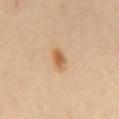No biopsy was performed on this lesion — it was imaged during a full skin examination and was not determined to be concerning. This image is a 15 mm lesion crop taken from a total-body photograph. This is a cross-polarized tile. A female subject aged 43–47. The lesion is on the mid back. Longest diameter approximately 2.5 mm.